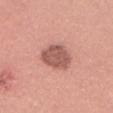Notes:
• follow-up · catalogued during a skin exam; not biopsied
• tile lighting · white-light
• subject · female, aged 33 to 37
• acquisition · total-body-photography crop, ~15 mm field of view
• TBP lesion metrics · a mean CIELAB color near L≈56 a*≈25 b*≈25, roughly 13 lightness units darker than nearby skin, and a lesion-to-skin contrast of about 8.5 (normalized; higher = more distinct); internal color variation of about 2 on a 0–10 scale and radial color variation of about 0.5
• diameter · ≈4.5 mm
• body site · the head or neck